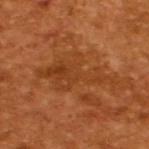Recorded during total-body skin imaging; not selected for excision or biopsy. A male patient aged approximately 65. The tile uses cross-polarized illumination. An algorithmic analysis of the crop reported an area of roughly 17 mm² and a shape eccentricity near 0.9. The analysis additionally found a border-irregularity rating of about 9/10, a color-variation rating of about 3.5/10, and a peripheral color-asymmetry measure near 1. A lesion tile, about 15 mm wide, cut from a 3D total-body photograph. The recorded lesion diameter is about 9 mm.